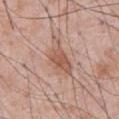biopsy status: imaged on a skin check; not biopsied
anatomic site: the front of the torso
illumination: white-light illumination
diameter: ~5 mm (longest diameter)
imaging modality: ~15 mm tile from a whole-body skin photo
patient: male, aged around 55
automated lesion analysis: an area of roughly 10 mm²; a border-irregularity rating of about 4.5/10, a color-variation rating of about 3.5/10, and peripheral color asymmetry of about 1; a detector confidence of about 100 out of 100 that the crop contains a lesion